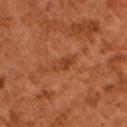Q: Was a biopsy performed?
A: no biopsy performed (imaged during a skin exam)
Q: How was this image acquired?
A: 15 mm crop, total-body photography
Q: Lesion size?
A: ≈3 mm
Q: Patient demographics?
A: male, in their mid- to late 60s
Q: Automated lesion metrics?
A: a footprint of about 4 mm² and a symmetry-axis asymmetry near 0.2; a mean CIELAB color near L≈41 a*≈28 b*≈36, a lesion–skin lightness drop of about 7, and a normalized lesion–skin contrast near 6; a border-irregularity index near 2.5/10, internal color variation of about 2 on a 0–10 scale, and a peripheral color-asymmetry measure near 0.5; a nevus-likeness score of about 0/100 and a detector confidence of about 100 out of 100 that the crop contains a lesion
Q: Illumination type?
A: cross-polarized